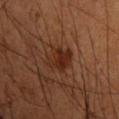Impression:
Part of a total-body skin-imaging series; this lesion was reviewed on a skin check and was not flagged for biopsy.
Clinical summary:
The total-body-photography lesion software estimated a color-variation rating of about 4/10 and radial color variation of about 1.5. Cropped from a whole-body photographic skin survey; the tile spans about 15 mm. The subject is a male aged approximately 50. On the left upper arm. Imaged with cross-polarized lighting.